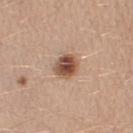Captured during whole-body skin photography for melanoma surveillance; the lesion was not biopsied. The subject is a female aged 43–47. The lesion-visualizer software estimated an average lesion color of about L≈50 a*≈21 b*≈29 (CIELAB), a lesion–skin lightness drop of about 16, and a normalized lesion–skin contrast near 11. And it measured a border-irregularity rating of about 1.5/10, a within-lesion color-variation index near 6/10, and peripheral color asymmetry of about 1.5. And it measured a classifier nevus-likeness of about 100/100 and a detector confidence of about 100 out of 100 that the crop contains a lesion. Measured at roughly 3 mm in maximum diameter. This is a white-light tile. Cropped from a total-body skin-imaging series; the visible field is about 15 mm. The lesion is located on the right thigh.